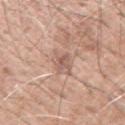Imaged during a routine full-body skin examination; the lesion was not biopsied and no histopathology is available.
An algorithmic analysis of the crop reported a nevus-likeness score of about 0/100 and lesion-presence confidence of about 55/100.
The tile uses white-light illumination.
The lesion is located on the right upper arm.
A male subject roughly 60 years of age.
This image is a 15 mm lesion crop taken from a total-body photograph.
Longest diameter approximately 4.5 mm.